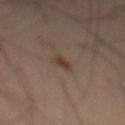notes = imaged on a skin check; not biopsied
subject = male, in their mid-50s
image source = 15 mm crop, total-body photography
site = the abdomen
illumination = cross-polarized